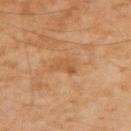notes: catalogued during a skin exam; not biopsied | lesion diameter: ≈2.5 mm | anatomic site: the right upper arm | lighting: cross-polarized illumination | patient: male, about 45 years old | automated metrics: an area of roughly 4.5 mm², an eccentricity of roughly 0.65, and a shape-asymmetry score of about 0.4 (0 = symmetric); an average lesion color of about L≈44 a*≈19 b*≈32 (CIELAB) and a lesion–skin lightness drop of about 5 | acquisition: ~15 mm tile from a whole-body skin photo.